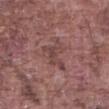Captured during whole-body skin photography for melanoma surveillance; the lesion was not biopsied.
Located on the abdomen.
The recorded lesion diameter is about 4 mm.
Cropped from a whole-body photographic skin survey; the tile spans about 15 mm.
The tile uses white-light illumination.
A male patient, roughly 75 years of age.